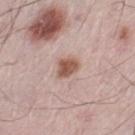Notes:
• notes: total-body-photography surveillance lesion; no biopsy
• illumination: white-light illumination
• acquisition: total-body-photography crop, ~15 mm field of view
• patient: male, roughly 55 years of age
• body site: the left thigh
• lesion size: about 2.5 mm